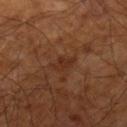Case summary:
* workup: total-body-photography surveillance lesion; no biopsy
* automated metrics: a color-variation rating of about 1/10 and a peripheral color-asymmetry measure near 0.5
* diameter: about 2.5 mm
* tile lighting: cross-polarized
* site: the leg
* imaging modality: ~15 mm crop, total-body skin-cancer survey
* patient: male, aged 58 to 62